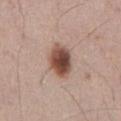Automated tile analysis of the lesion measured a border-irregularity rating of about 1.5/10, internal color variation of about 6.5 on a 0–10 scale, and a peripheral color-asymmetry measure near 2. From the abdomen. The tile uses white-light illumination. Approximately 4.5 mm at its widest. A lesion tile, about 15 mm wide, cut from a 3D total-body photograph. The patient is a male in their mid-50s.